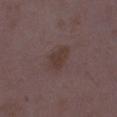The lesion was photographed on a routine skin check and not biopsied; there is no pathology result.
Captured under white-light illumination.
The patient is a female aged 33–37.
A region of skin cropped from a whole-body photographic capture, roughly 15 mm wide.
The lesion is on the right thigh.
About 3.5 mm across.